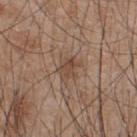| key | value |
|---|---|
| biopsy status | no biopsy performed (imaged during a skin exam) |
| location | the upper back |
| automated metrics | a lesion area of about 4.5 mm² and two-axis asymmetry of about 0.3; a border-irregularity index near 3.5/10, a within-lesion color-variation index near 3/10, and a peripheral color-asymmetry measure near 1 |
| image source | 15 mm crop, total-body photography |
| subject | male, in their mid- to late 40s |
| illumination | white-light illumination |